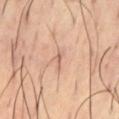{"biopsy_status": "not biopsied; imaged during a skin examination", "site": "abdomen", "image": {"source": "total-body photography crop", "field_of_view_mm": 15}, "patient": {"sex": "male", "age_approx": 40}}